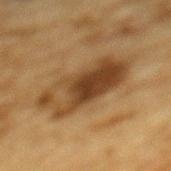A male patient, aged around 85 or older. The tile uses cross-polarized illumination. The lesion is on the mid back. A 15 mm close-up tile from a total-body photography series done for melanoma screening. About 8.5 mm across.